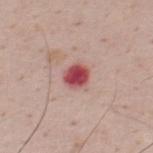Part of a total-body skin-imaging series; this lesion was reviewed on a skin check and was not flagged for biopsy.
Measured at roughly 2.5 mm in maximum diameter.
An algorithmic analysis of the crop reported a color-variation rating of about 3.5/10 and peripheral color asymmetry of about 1.
A male patient aged 33–37.
Imaged with white-light lighting.
Located on the upper back.
A region of skin cropped from a whole-body photographic capture, roughly 15 mm wide.